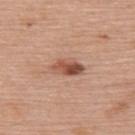Notes:
– follow-up: no biopsy performed (imaged during a skin exam)
– automated metrics: a detector confidence of about 100 out of 100 that the crop contains a lesion
– tile lighting: white-light
– lesion size: ~4 mm (longest diameter)
– body site: the upper back
– patient: female, aged 58–62
– image: ~15 mm crop, total-body skin-cancer survey